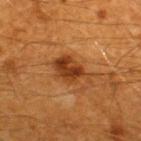| field | value |
|---|---|
| follow-up | catalogued during a skin exam; not biopsied |
| body site | the upper back |
| image | ~15 mm tile from a whole-body skin photo |
| patient | male, aged 58 to 62 |
| lesion size | ~4.5 mm (longest diameter) |